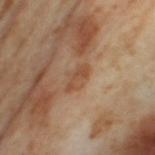The lesion was tiled from a total-body skin photograph and was not biopsied.
The subject is a female aged 53–57.
A close-up tile cropped from a whole-body skin photograph, about 15 mm across.
The tile uses cross-polarized illumination.
The lesion is located on the left thigh.
Automated tile analysis of the lesion measured an average lesion color of about L≈50 a*≈19 b*≈33 (CIELAB), about 7 CIELAB-L* units darker than the surrounding skin, and a normalized lesion–skin contrast near 6. The software also gave an automated nevus-likeness rating near 0 out of 100 and a lesion-detection confidence of about 100/100.